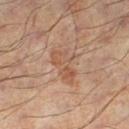| feature | finding |
|---|---|
| biopsy status | total-body-photography surveillance lesion; no biopsy |
| illumination | cross-polarized illumination |
| lesion diameter | about 4.5 mm |
| image source | ~15 mm crop, total-body skin-cancer survey |
| location | the left lower leg |
| subject | male, approximately 55 years of age |